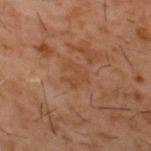– notes · no biopsy performed (imaged during a skin exam)
– patient · male, aged approximately 60
– image source · ~15 mm tile from a whole-body skin photo
– location · the upper back